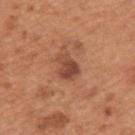Notes:
– acquisition — ~15 mm crop, total-body skin-cancer survey
– diameter — ≈3 mm
– anatomic site — the upper back
– subject — male, aged approximately 65
– image-analysis metrics — a shape eccentricity near 0.55 and a symmetry-axis asymmetry near 0.35; a nevus-likeness score of about 25/100 and a lesion-detection confidence of about 100/100